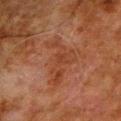{"biopsy_status": "not biopsied; imaged during a skin examination", "image": {"source": "total-body photography crop", "field_of_view_mm": 15}, "patient": {"sex": "male", "age_approx": 80}, "lighting": "cross-polarized", "site": "arm"}